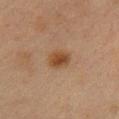Q: Is there a histopathology result?
A: no biopsy performed (imaged during a skin exam)
Q: Lesion location?
A: the chest
Q: How was this image acquired?
A: 15 mm crop, total-body photography
Q: What are the patient's age and sex?
A: female, aged 38 to 42